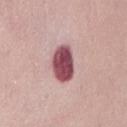biopsy status: total-body-photography surveillance lesion; no biopsy
TBP lesion metrics: a border-irregularity rating of about 2/10 and a within-lesion color-variation index near 4.5/10
anatomic site: the abdomen
image source: total-body-photography crop, ~15 mm field of view
subject: female, roughly 50 years of age